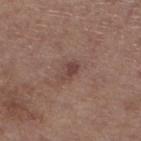biopsy_status: not biopsied; imaged during a skin examination
lighting: white-light
patient:
  sex: female
  age_approx: 65
image:
  source: total-body photography crop
  field_of_view_mm: 15
site: leg